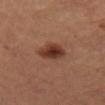Part of a total-body skin-imaging series; this lesion was reviewed on a skin check and was not flagged for biopsy. The patient is female. Cropped from a total-body skin-imaging series; the visible field is about 15 mm. From the abdomen. Approximately 4 mm at its widest.